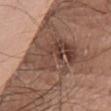TBP lesion metrics = a shape eccentricity near 0.4 and two-axis asymmetry of about 0.4; an automated nevus-likeness rating near 0 out of 100 and lesion-presence confidence of about 85/100 | subject = male, roughly 65 years of age | site = the chest | imaging modality = total-body-photography crop, ~15 mm field of view | size = ~6.5 mm (longest diameter).